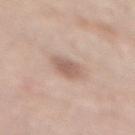Clinical impression: Part of a total-body skin-imaging series; this lesion was reviewed on a skin check and was not flagged for biopsy. Context: From the mid back. A female subject, roughly 65 years of age. Imaged with white-light lighting. The lesion's longest dimension is about 3 mm. This image is a 15 mm lesion crop taken from a total-body photograph. Automated tile analysis of the lesion measured a lesion color around L≈59 a*≈17 b*≈26 in CIELAB, roughly 10 lightness units darker than nearby skin, and a normalized border contrast of about 7. It also reported a nevus-likeness score of about 30/100 and a detector confidence of about 100 out of 100 that the crop contains a lesion.